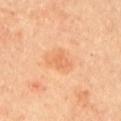The lesion was photographed on a routine skin check and not biopsied; there is no pathology result.
The subject is a male aged approximately 65.
This is a cross-polarized tile.
From the left upper arm.
An algorithmic analysis of the crop reported a lesion area of about 4.5 mm², an eccentricity of roughly 0.6, and a shape-asymmetry score of about 0.45 (0 = symmetric). It also reported a classifier nevus-likeness of about 25/100 and lesion-presence confidence of about 100/100.
A lesion tile, about 15 mm wide, cut from a 3D total-body photograph.